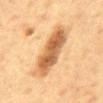workup: no biopsy performed (imaged during a skin exam) | subject: male, in their 60s | location: the abdomen | size: about 7.5 mm | image source: ~15 mm tile from a whole-body skin photo | tile lighting: cross-polarized illumination.